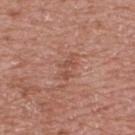Case summary:
- notes: no biopsy performed (imaged during a skin exam)
- patient: male, aged 68–72
- lighting: white-light
- automated metrics: a lesion area of about 3.5 mm² and a symmetry-axis asymmetry near 0.5; a border-irregularity rating of about 7.5/10; a nevus-likeness score of about 0/100 and a lesion-detection confidence of about 100/100
- anatomic site: the upper back
- diameter: ~3 mm (longest diameter)
- image source: ~15 mm tile from a whole-body skin photo